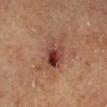Case summary:
• notes · no biopsy performed (imaged during a skin exam)
• automated metrics · an area of roughly 16 mm², an eccentricity of roughly 0.8, and two-axis asymmetry of about 0.35
• illumination · cross-polarized illumination
• site · the right lower leg
• patient · male, about 85 years old or older
• lesion size · ~6.5 mm (longest diameter)
• image · total-body-photography crop, ~15 mm field of view